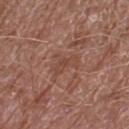follow-up = imaged on a skin check; not biopsied | subject = male, approximately 60 years of age | imaging modality = total-body-photography crop, ~15 mm field of view | body site = the left lower leg.